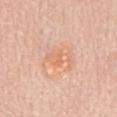Case summary:
– follow-up — catalogued during a skin exam; not biopsied
– lighting — white-light illumination
– patient — female, aged 63–67
– image — ~15 mm crop, total-body skin-cancer survey
– diameter — ≈3.5 mm
– TBP lesion metrics — a shape eccentricity near 0.8 and a symmetry-axis asymmetry near 0.35
– body site — the back